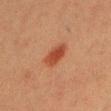Image and clinical context: An algorithmic analysis of the crop reported a nevus-likeness score of about 100/100 and lesion-presence confidence of about 100/100. Cropped from a total-body skin-imaging series; the visible field is about 15 mm. The patient is a male in their 40s. This is a cross-polarized tile. The lesion is on the front of the torso.